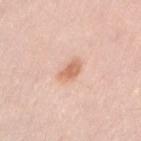Imaged during a routine full-body skin examination; the lesion was not biopsied and no histopathology is available. The tile uses white-light illumination. Cropped from a total-body skin-imaging series; the visible field is about 15 mm. A female patient, aged around 45. On the right upper arm. Approximately 3 mm at its widest.